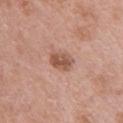Assessment: Captured during whole-body skin photography for melanoma surveillance; the lesion was not biopsied. Acquisition and patient details: A female patient, in their mid- to late 60s. Located on the upper back. A roughly 15 mm field-of-view crop from a total-body skin photograph.